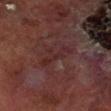Impression: The lesion was tiled from a total-body skin photograph and was not biopsied. Acquisition and patient details: A male patient, aged 68–72. On the right lower leg. Automated image analysis of the tile measured a border-irregularity rating of about 8.5/10, internal color variation of about 0 on a 0–10 scale, and peripheral color asymmetry of about 0. The analysis additionally found a classifier nevus-likeness of about 0/100 and lesion-presence confidence of about 85/100. Cropped from a total-body skin-imaging series; the visible field is about 15 mm.